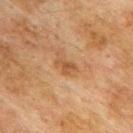Impression: The lesion was tiled from a total-body skin photograph and was not biopsied. Background: A 15 mm close-up extracted from a 3D total-body photography capture. Approximately 2.5 mm at its widest. The total-body-photography lesion software estimated a lesion area of about 3.5 mm², an eccentricity of roughly 0.8, and two-axis asymmetry of about 0.45. The patient is a male approximately 75 years of age. The tile uses cross-polarized illumination. Located on the upper back.